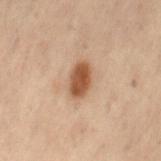Q: Was this lesion biopsied?
A: no biopsy performed (imaged during a skin exam)
Q: Automated lesion metrics?
A: a footprint of about 7 mm², an eccentricity of roughly 0.8, and a shape-asymmetry score of about 0.15 (0 = symmetric); a border-irregularity rating of about 1.5/10, internal color variation of about 3 on a 0–10 scale, and radial color variation of about 1; an automated nevus-likeness rating near 100 out of 100 and a lesion-detection confidence of about 100/100
Q: How was this image acquired?
A: 15 mm crop, total-body photography
Q: Illumination type?
A: cross-polarized
Q: Who is the patient?
A: female, in their mid- to late 50s
Q: How large is the lesion?
A: about 3.5 mm
Q: Lesion location?
A: the lower back